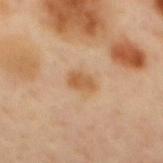Clinical impression:
This lesion was catalogued during total-body skin photography and was not selected for biopsy.
Clinical summary:
Cropped from a total-body skin-imaging series; the visible field is about 15 mm. A male subject, about 40 years old. The lesion is on the mid back.